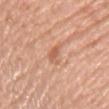Clinical impression:
No biopsy was performed on this lesion — it was imaged during a full skin examination and was not determined to be concerning.
Context:
The lesion is located on the chest. Cropped from a whole-body photographic skin survey; the tile spans about 15 mm. Measured at roughly 3 mm in maximum diameter. Imaged with white-light lighting. A male subject approximately 60 years of age. Automated tile analysis of the lesion measured a lesion color around L≈60 a*≈25 b*≈34 in CIELAB and a lesion-to-skin contrast of about 6 (normalized; higher = more distinct). And it measured a detector confidence of about 100 out of 100 that the crop contains a lesion.